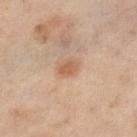Imaged during a routine full-body skin examination; the lesion was not biopsied and no histopathology is available. Located on the left lower leg. A female subject in their 50s. Cropped from a total-body skin-imaging series; the visible field is about 15 mm.